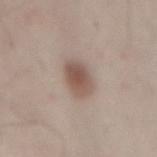No biopsy was performed on this lesion — it was imaged during a full skin examination and was not determined to be concerning.
Imaged with white-light lighting.
A male subject, aged 53 to 57.
On the mid back.
An algorithmic analysis of the crop reported a lesion color around L≈53 a*≈16 b*≈24 in CIELAB, a lesion–skin lightness drop of about 12, and a normalized border contrast of about 8.5. The software also gave a nevus-likeness score of about 100/100 and a lesion-detection confidence of about 100/100.
The recorded lesion diameter is about 4 mm.
A 15 mm close-up tile from a total-body photography series done for melanoma screening.